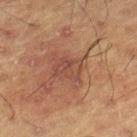<tbp_lesion>
<biopsy_status>not biopsied; imaged during a skin examination</biopsy_status>
<patient>
  <sex>male</sex>
  <age_approx>60</age_approx>
</patient>
<site>right lower leg</site>
<image>
  <source>total-body photography crop</source>
  <field_of_view_mm>15</field_of_view_mm>
</image>
</tbp_lesion>